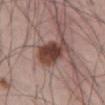{"biopsy_status": "not biopsied; imaged during a skin examination", "site": "abdomen", "patient": {"sex": "male", "age_approx": 55}, "image": {"source": "total-body photography crop", "field_of_view_mm": 15}}